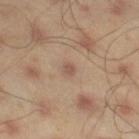notes — total-body-photography surveillance lesion; no biopsy | site — the right thigh | subject — male, approximately 45 years of age | lesion diameter — about 1.5 mm | automated lesion analysis — a color-variation rating of about 1/10 | acquisition — ~15 mm crop, total-body skin-cancer survey | illumination — cross-polarized illumination.